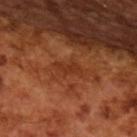The lesion was photographed on a routine skin check and not biopsied; there is no pathology result.
The patient is a male aged around 65.
Imaged with cross-polarized lighting.
A region of skin cropped from a whole-body photographic capture, roughly 15 mm wide.
The lesion-visualizer software estimated border irregularity of about 6.5 on a 0–10 scale and a within-lesion color-variation index near 0/10. The software also gave a classifier nevus-likeness of about 0/100 and lesion-presence confidence of about 95/100.